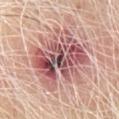Findings:
- follow-up: imaged on a skin check; not biopsied
- image-analysis metrics: an area of roughly 40 mm², an outline eccentricity of about 0.65 (0 = round, 1 = elongated), and a symmetry-axis asymmetry near 0.25
- image: ~15 mm tile from a whole-body skin photo
- patient: male, aged 63–67
- size: ≈9 mm
- site: the left upper arm